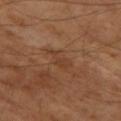The lesion was tiled from a total-body skin photograph and was not biopsied.
The tile uses cross-polarized illumination.
A 15 mm crop from a total-body photograph taken for skin-cancer surveillance.
Automated tile analysis of the lesion measured a border-irregularity index near 6/10 and radial color variation of about 0.5. And it measured a nevus-likeness score of about 0/100.
A male patient, about 65 years old.
Measured at roughly 3.5 mm in maximum diameter.